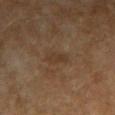follow-up — no biopsy performed (imaged during a skin exam); lighting — cross-polarized illumination; diameter — ≈3 mm; site — the right upper arm; patient — female, about 60 years old; image — ~15 mm crop, total-body skin-cancer survey.